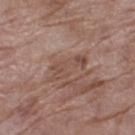Imaged during a routine full-body skin examination; the lesion was not biopsied and no histopathology is available. The tile uses white-light illumination. From the left thigh. A region of skin cropped from a whole-body photographic capture, roughly 15 mm wide. The patient is a female approximately 70 years of age.